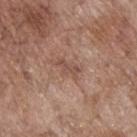No biopsy was performed on this lesion — it was imaged during a full skin examination and was not determined to be concerning.
On the upper back.
Measured at roughly 3 mm in maximum diameter.
The lesion-visualizer software estimated a lesion color around L≈50 a*≈19 b*≈26 in CIELAB and a normalized border contrast of about 6. The analysis additionally found a classifier nevus-likeness of about 0/100 and lesion-presence confidence of about 100/100.
A male subject, aged 68 to 72.
This is a white-light tile.
A region of skin cropped from a whole-body photographic capture, roughly 15 mm wide.